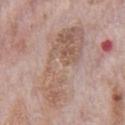Impression:
No biopsy was performed on this lesion — it was imaged during a full skin examination and was not determined to be concerning.
Background:
A close-up tile cropped from a whole-body skin photograph, about 15 mm across. Longest diameter approximately 10.5 mm. A male patient approximately 70 years of age. The lesion-visualizer software estimated an average lesion color of about L≈58 a*≈17 b*≈26 (CIELAB), about 8 CIELAB-L* units darker than the surrounding skin, and a normalized border contrast of about 6. The analysis additionally found a border-irregularity index near 8/10, a within-lesion color-variation index near 5/10, and a peripheral color-asymmetry measure near 2. Imaged with white-light lighting. From the chest.